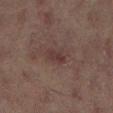The lesion was photographed on a routine skin check and not biopsied; there is no pathology result.
The lesion is located on the left lower leg.
The subject is a female aged 68 to 72.
The recorded lesion diameter is about 2.5 mm.
A region of skin cropped from a whole-body photographic capture, roughly 15 mm wide.
The tile uses cross-polarized illumination.
The lesion-visualizer software estimated a lesion color around L≈28 a*≈16 b*≈15 in CIELAB, about 6 CIELAB-L* units darker than the surrounding skin, and a normalized border contrast of about 6.5. The software also gave a border-irregularity index near 3.5/10, internal color variation of about 2 on a 0–10 scale, and peripheral color asymmetry of about 0.5. The software also gave an automated nevus-likeness rating near 15 out of 100.